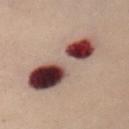{"biopsy_status": "not biopsied; imaged during a skin examination", "lighting": "cross-polarized", "site": "front of the torso", "lesion_size": {"long_diameter_mm_approx": 10.0}, "patient": {"sex": "female", "age_approx": 50}, "image": {"source": "total-body photography crop", "field_of_view_mm": 15}}